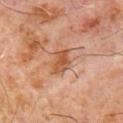Q: Was a biopsy performed?
A: imaged on a skin check; not biopsied
Q: What is the imaging modality?
A: ~15 mm tile from a whole-body skin photo
Q: What lighting was used for the tile?
A: cross-polarized illumination
Q: What is the anatomic site?
A: the chest
Q: What is the lesion's diameter?
A: ≈3.5 mm
Q: Who is the patient?
A: male, aged 58 to 62